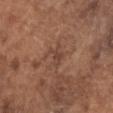| feature | finding |
|---|---|
| notes | no biopsy performed (imaged during a skin exam) |
| patient | male, about 75 years old |
| diameter | ≈3 mm |
| illumination | white-light |
| imaging modality | ~15 mm crop, total-body skin-cancer survey |
| body site | the head or neck |
| automated metrics | an average lesion color of about L≈42 a*≈20 b*≈26 (CIELAB) and a lesion–skin lightness drop of about 6; an automated nevus-likeness rating near 0 out of 100 and a lesion-detection confidence of about 90/100 |